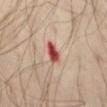Findings:
• biopsy status: no biopsy performed (imaged during a skin exam)
• lesion size: ~3 mm (longest diameter)
• imaging modality: total-body-photography crop, ~15 mm field of view
• subject: male, aged 43–47
• site: the abdomen